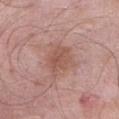{"biopsy_status": "not biopsied; imaged during a skin examination", "lighting": "white-light", "lesion_size": {"long_diameter_mm_approx": 4.0}, "automated_metrics": {"cielab_L": 54, "cielab_a": 22, "cielab_b": 26, "vs_skin_darker_L": 8.0, "vs_skin_contrast_norm": 6.0}, "patient": {"sex": "male", "age_approx": 55}, "site": "abdomen", "image": {"source": "total-body photography crop", "field_of_view_mm": 15}}